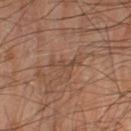| field | value |
|---|---|
| illumination | cross-polarized |
| lesion size | ~3.5 mm (longest diameter) |
| site | the left thigh |
| subject | male, aged around 65 |
| image-analysis metrics | an area of roughly 3.5 mm², an eccentricity of roughly 0.9, and a shape-asymmetry score of about 0.7 (0 = symmetric) |
| image source | total-body-photography crop, ~15 mm field of view |